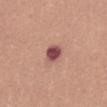Clinical impression: Recorded during total-body skin imaging; not selected for excision or biopsy. Context: An algorithmic analysis of the crop reported a lesion color around L≈49 a*≈27 b*≈21 in CIELAB, about 16 CIELAB-L* units darker than the surrounding skin, and a normalized border contrast of about 11.5. The analysis additionally found a within-lesion color-variation index near 3.5/10 and a peripheral color-asymmetry measure near 1. And it measured a detector confidence of about 100 out of 100 that the crop contains a lesion. Measured at roughly 2.5 mm in maximum diameter. This is a white-light tile. A 15 mm close-up extracted from a 3D total-body photography capture. A female subject, aged around 35. The lesion is on the abdomen.